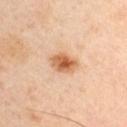Assessment: The lesion was tiled from a total-body skin photograph and was not biopsied. Background: From the arm. A male patient in their mid-40s. A 15 mm close-up tile from a total-body photography series done for melanoma screening. The tile uses cross-polarized illumination. Longest diameter approximately 3.5 mm.